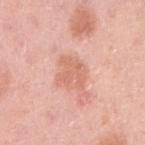{
  "lesion_size": {
    "long_diameter_mm_approx": 3.5
  },
  "lighting": "white-light",
  "image": {
    "source": "total-body photography crop",
    "field_of_view_mm": 15
  },
  "site": "right upper arm",
  "patient": {
    "sex": "female",
    "age_approx": 30
  }
}